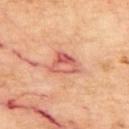Recorded during total-body skin imaging; not selected for excision or biopsy.
A roughly 15 mm field-of-view crop from a total-body skin photograph.
The subject is a male roughly 70 years of age.
The recorded lesion diameter is about 3.5 mm.
From the upper back.
The tile uses cross-polarized illumination.